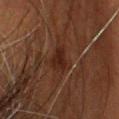{"lesion_size": {"long_diameter_mm_approx": 3.0}, "image": {"source": "total-body photography crop", "field_of_view_mm": 15}, "patient": {"sex": "female", "age_approx": 55}, "lighting": "cross-polarized", "site": "head or neck"}